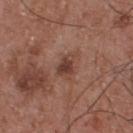{
  "biopsy_status": "not biopsied; imaged during a skin examination",
  "patient": {
    "sex": "male",
    "age_approx": 55
  },
  "lesion_size": {
    "long_diameter_mm_approx": 2.5
  },
  "automated_metrics": {
    "area_mm2_approx": 4.5,
    "cielab_L": 40,
    "cielab_a": 21,
    "cielab_b": 25,
    "vs_skin_contrast_norm": 8.0
  },
  "site": "chest",
  "image": {
    "source": "total-body photography crop",
    "field_of_view_mm": 15
  },
  "lighting": "white-light"
}